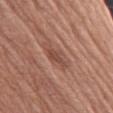follow-up: catalogued during a skin exam; not biopsied
imaging modality: ~15 mm tile from a whole-body skin photo
body site: the right upper arm
subject: female, aged 73–77
TBP lesion metrics: an area of roughly 4 mm² and an outline eccentricity of about 0.8 (0 = round, 1 = elongated); border irregularity of about 3.5 on a 0–10 scale, internal color variation of about 1.5 on a 0–10 scale, and radial color variation of about 0.5; an automated nevus-likeness rating near 0 out of 100 and lesion-presence confidence of about 95/100